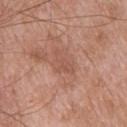No biopsy was performed on this lesion — it was imaged during a full skin examination and was not determined to be concerning.
The patient is a female aged 63–67.
Imaged with white-light lighting.
Measured at roughly 3.5 mm in maximum diameter.
A 15 mm close-up tile from a total-body photography series done for melanoma screening.
From the upper back.